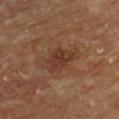Captured during whole-body skin photography for melanoma surveillance; the lesion was not biopsied. The lesion is on the front of the torso. A 15 mm close-up tile from a total-body photography series done for melanoma screening. The patient is a female about 80 years old.